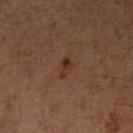Imaged during a routine full-body skin examination; the lesion was not biopsied and no histopathology is available.
A 15 mm close-up tile from a total-body photography series done for melanoma screening.
The patient is a female aged 53–57.
From the left forearm.
Automated image analysis of the tile measured a lesion color around L≈26 a*≈16 b*≈23 in CIELAB, a lesion–skin lightness drop of about 6, and a lesion-to-skin contrast of about 7 (normalized; higher = more distinct). It also reported a nevus-likeness score of about 40/100 and a detector confidence of about 100 out of 100 that the crop contains a lesion.